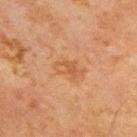<case>
  <biopsy_status>not biopsied; imaged during a skin examination</biopsy_status>
  <lighting>cross-polarized</lighting>
  <lesion_size>
    <long_diameter_mm_approx>3.5</long_diameter_mm_approx>
  </lesion_size>
  <patient>
    <sex>male</sex>
    <age_approx>65</age_approx>
  </patient>
  <site>front of the torso</site>
  <image>
    <source>total-body photography crop</source>
    <field_of_view_mm>15</field_of_view_mm>
  </image>
</case>